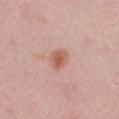• notes · catalogued during a skin exam; not biopsied
• imaging modality · total-body-photography crop, ~15 mm field of view
• body site · the right thigh
• lighting · white-light illumination
• lesion size · ≈2.5 mm
• patient · male, roughly 40 years of age